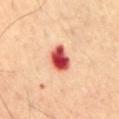Q: Is there a histopathology result?
A: catalogued during a skin exam; not biopsied
Q: How was the tile lit?
A: cross-polarized
Q: What is the anatomic site?
A: the abdomen
Q: How large is the lesion?
A: ~3.5 mm (longest diameter)
Q: How was this image acquired?
A: total-body-photography crop, ~15 mm field of view
Q: Who is the patient?
A: male, aged 68 to 72